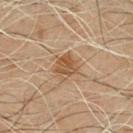Acquisition and patient details: The patient is a male aged 53 to 57. The lesion is located on the chest. Cropped from a total-body skin-imaging series; the visible field is about 15 mm.